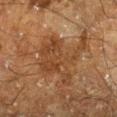Impression:
Recorded during total-body skin imaging; not selected for excision or biopsy.
Context:
A 15 mm crop from a total-body photograph taken for skin-cancer surveillance. Measured at roughly 6.5 mm in maximum diameter. Captured under cross-polarized illumination. The lesion is on the right lower leg. The subject is a male aged 58 to 62.